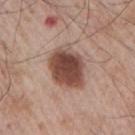Q: Was a biopsy performed?
A: total-body-photography surveillance lesion; no biopsy
Q: What is the anatomic site?
A: the right upper arm
Q: What did automated image analysis measure?
A: a classifier nevus-likeness of about 90/100 and a lesion-detection confidence of about 100/100
Q: What kind of image is this?
A: ~15 mm crop, total-body skin-cancer survey
Q: Lesion size?
A: ~5 mm (longest diameter)
Q: What lighting was used for the tile?
A: white-light illumination
Q: Patient demographics?
A: male, aged 63–67